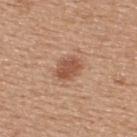Part of a total-body skin-imaging series; this lesion was reviewed on a skin check and was not flagged for biopsy. Approximately 3 mm at its widest. A close-up tile cropped from a whole-body skin photograph, about 15 mm across. The lesion is on the upper back. The patient is a female approximately 45 years of age. Captured under white-light illumination. Automated tile analysis of the lesion measured a footprint of about 5.5 mm², an outline eccentricity of about 0.7 (0 = round, 1 = elongated), and a shape-asymmetry score of about 0.15 (0 = symmetric). It also reported a border-irregularity rating of about 1.5/10, internal color variation of about 2.5 on a 0–10 scale, and peripheral color asymmetry of about 1.